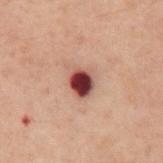  biopsy_status: not biopsied; imaged during a skin examination
  lighting: cross-polarized
  site: abdomen
  lesion_size:
    long_diameter_mm_approx: 3.5
  image:
    source: total-body photography crop
    field_of_view_mm: 15
  patient:
    sex: male
    age_approx: 60
  automated_metrics:
    cielab_L: 35
    cielab_a: 21
    cielab_b: 20
    vs_skin_darker_L: 15.0
    vs_skin_contrast_norm: 13.0
    color_variation_0_10: 8.5
    peripheral_color_asymmetry: 2.5